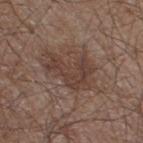Q: Is there a histopathology result?
A: imaged on a skin check; not biopsied
Q: What lighting was used for the tile?
A: white-light illumination
Q: What are the patient's age and sex?
A: male, aged around 60
Q: Automated lesion metrics?
A: an area of roughly 13 mm², an eccentricity of roughly 0.75, and a symmetry-axis asymmetry near 0.6; about 8 CIELAB-L* units darker than the surrounding skin; a classifier nevus-likeness of about 5/100
Q: Where on the body is the lesion?
A: the right thigh
Q: How was this image acquired?
A: 15 mm crop, total-body photography
Q: How large is the lesion?
A: about 5.5 mm